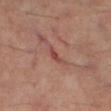Clinical summary:
From the left thigh. A male subject, about 60 years old. A close-up tile cropped from a whole-body skin photograph, about 15 mm across.